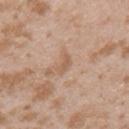Impression:
Recorded during total-body skin imaging; not selected for excision or biopsy.
Background:
A 15 mm crop from a total-body photograph taken for skin-cancer surveillance. The lesion is on the arm. The subject is a female approximately 25 years of age.